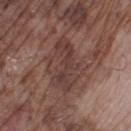Part of a total-body skin-imaging series; this lesion was reviewed on a skin check and was not flagged for biopsy. On the right lower leg. This is a white-light tile. The recorded lesion diameter is about 6.5 mm. A region of skin cropped from a whole-body photographic capture, roughly 15 mm wide. An algorithmic analysis of the crop reported roughly 8 lightness units darker than nearby skin. The software also gave a border-irregularity rating of about 5.5/10 and internal color variation of about 3.5 on a 0–10 scale. The subject is a male aged around 75.